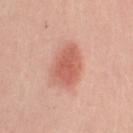Captured during whole-body skin photography for melanoma surveillance; the lesion was not biopsied.
The lesion is on the left upper arm.
A close-up tile cropped from a whole-body skin photograph, about 15 mm across.
A female subject, in their 50s.